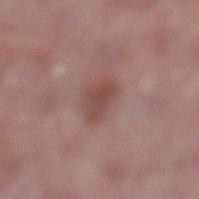• follow-up — no biopsy performed (imaged during a skin exam)